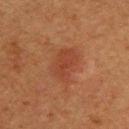workup: catalogued during a skin exam; not biopsied
lighting: cross-polarized
lesion diameter: about 4 mm
patient: female, aged around 40
TBP lesion metrics: an eccentricity of roughly 0.75 and two-axis asymmetry of about 0.2; a lesion color around L≈36 a*≈23 b*≈29 in CIELAB and roughly 6 lightness units darker than nearby skin; a border-irregularity rating of about 2/10, a within-lesion color-variation index near 2.5/10, and a peripheral color-asymmetry measure near 1
site: the upper back
acquisition: total-body-photography crop, ~15 mm field of view